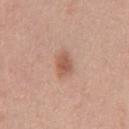Findings:
- notes · catalogued during a skin exam; not biopsied
- subject · female, in their 40s
- acquisition · 15 mm crop, total-body photography
- anatomic site · the chest